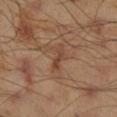biopsy status: catalogued during a skin exam; not biopsied
location: the right lower leg
acquisition: ~15 mm tile from a whole-body skin photo
subject: male, aged 43–47